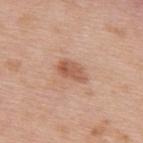The lesion was photographed on a routine skin check and not biopsied; there is no pathology result. About 3.5 mm across. Imaged with white-light lighting. A 15 mm close-up extracted from a 3D total-body photography capture. A female subject in their 40s. Located on the upper back.